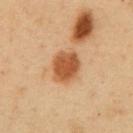Assessment: No biopsy was performed on this lesion — it was imaged during a full skin examination and was not determined to be concerning. Image and clinical context: Cropped from a whole-body photographic skin survey; the tile spans about 15 mm. The lesion's longest dimension is about 4 mm. The lesion is located on the abdomen. A male subject, aged approximately 50.